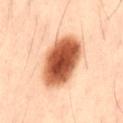biopsy status = total-body-photography surveillance lesion; no biopsy | subject = male, aged 38 to 42 | image source = ~15 mm tile from a whole-body skin photo | site = the lower back | image-analysis metrics = an automated nevus-likeness rating near 100 out of 100.